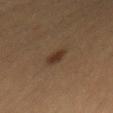| key | value |
|---|---|
| workup | no biopsy performed (imaged during a skin exam) |
| image source | ~15 mm tile from a whole-body skin photo |
| site | the mid back |
| patient | male, about 60 years old |
| lighting | cross-polarized |
| size | ≈3 mm |